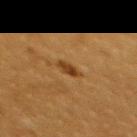Clinical summary: The lesion-visualizer software estimated an average lesion color of about L≈34 a*≈19 b*≈34 (CIELAB) and a lesion-to-skin contrast of about 8.5 (normalized; higher = more distinct). It also reported a classifier nevus-likeness of about 95/100 and a lesion-detection confidence of about 100/100. Located on the upper back. Imaged with cross-polarized lighting. A female subject aged approximately 55. A 15 mm crop from a total-body photograph taken for skin-cancer surveillance.